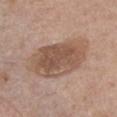No biopsy was performed on this lesion — it was imaged during a full skin examination and was not determined to be concerning.
Automated image analysis of the tile measured an area of roughly 28 mm² and a shape-asymmetry score of about 0.2 (0 = symmetric). The software also gave border irregularity of about 2.5 on a 0–10 scale, a within-lesion color-variation index near 4.5/10, and a peripheral color-asymmetry measure near 1.5. The analysis additionally found a nevus-likeness score of about 25/100 and lesion-presence confidence of about 100/100.
A male patient about 75 years old.
Captured under white-light illumination.
On the chest.
A close-up tile cropped from a whole-body skin photograph, about 15 mm across.
The lesion's longest dimension is about 8 mm.